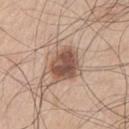This is a white-light tile. The total-body-photography lesion software estimated a lesion area of about 11 mm², an eccentricity of roughly 0.6, and a symmetry-axis asymmetry near 0.15. The analysis additionally found an automated nevus-likeness rating near 95 out of 100 and a lesion-detection confidence of about 100/100. A male patient, roughly 70 years of age. A close-up tile cropped from a whole-body skin photograph, about 15 mm across. Approximately 4 mm at its widest. Located on the left upper arm.